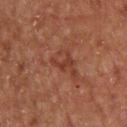biopsy_status: not biopsied; imaged during a skin examination
image:
  source: total-body photography crop
  field_of_view_mm: 15
lesion_size:
  long_diameter_mm_approx: 3.0
patient:
  sex: male
  age_approx: 55
lighting: cross-polarized
site: upper back